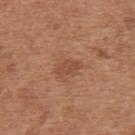Q: Was a biopsy performed?
A: total-body-photography surveillance lesion; no biopsy
Q: How was the tile lit?
A: white-light illumination
Q: Automated lesion metrics?
A: a lesion area of about 3.5 mm² and a shape-asymmetry score of about 0.2 (0 = symmetric); about 7 CIELAB-L* units darker than the surrounding skin and a lesion-to-skin contrast of about 5.5 (normalized; higher = more distinct); a classifier nevus-likeness of about 40/100 and a lesion-detection confidence of about 100/100
Q: What is the lesion's diameter?
A: ≈2.5 mm
Q: What is the imaging modality?
A: ~15 mm crop, total-body skin-cancer survey
Q: What are the patient's age and sex?
A: female, roughly 40 years of age
Q: Lesion location?
A: the back